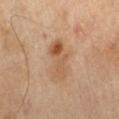{"biopsy_status": "not biopsied; imaged during a skin examination"}